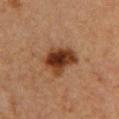follow-up = no biopsy performed (imaged during a skin exam)
body site = the chest
image = 15 mm crop, total-body photography
patient = female, about 40 years old